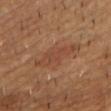Notes:
* workup · catalogued during a skin exam; not biopsied
* acquisition · total-body-photography crop, ~15 mm field of view
* anatomic site · the chest
* subject · male, aged 83 or older